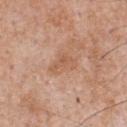Notes:
* workup · no biopsy performed (imaged during a skin exam)
* patient · male, roughly 65 years of age
* body site · the chest
* size · ~3.5 mm (longest diameter)
* illumination · white-light illumination
* image-analysis metrics · a within-lesion color-variation index near 3/10 and peripheral color asymmetry of about 1; a nevus-likeness score of about 0/100 and a detector confidence of about 100 out of 100 that the crop contains a lesion
* image source · ~15 mm tile from a whole-body skin photo